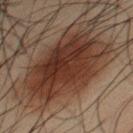notes = no biopsy performed (imaged during a skin exam) | subject = male, in their 60s | automated metrics = an average lesion color of about L≈28 a*≈16 b*≈22 (CIELAB), roughly 10 lightness units darker than nearby skin, and a normalized lesion–skin contrast near 11; a border-irregularity rating of about 3/10; a lesion-detection confidence of about 100/100 | image source = ~15 mm crop, total-body skin-cancer survey | tile lighting = cross-polarized | body site = the chest.